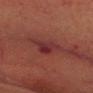{"biopsy_status": "not biopsied; imaged during a skin examination", "automated_metrics": {"area_mm2_approx": 9.0, "eccentricity": 0.8, "shape_asymmetry": 0.45, "cielab_L": 26, "cielab_a": 23, "cielab_b": 19, "vs_skin_darker_L": 6.0, "vs_skin_contrast_norm": 7.0, "peripheral_color_asymmetry": 1.0}, "lesion_size": {"long_diameter_mm_approx": 5.0}, "image": {"source": "total-body photography crop", "field_of_view_mm": 15}, "site": "head or neck", "patient": {"sex": "male", "age_approx": 70}, "lighting": "cross-polarized"}